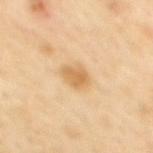Recorded during total-body skin imaging; not selected for excision or biopsy.
Automated image analysis of the tile measured a lesion area of about 6 mm², an eccentricity of roughly 0.75, and a symmetry-axis asymmetry near 0.2. The analysis additionally found an average lesion color of about L≈68 a*≈18 b*≈43 (CIELAB) and a normalized lesion–skin contrast near 7. The software also gave an automated nevus-likeness rating near 65 out of 100 and a lesion-detection confidence of about 100/100.
A female subject, aged 43 to 47.
Measured at roughly 3.5 mm in maximum diameter.
Imaged with cross-polarized lighting.
A 15 mm close-up extracted from a 3D total-body photography capture.
Located on the upper back.